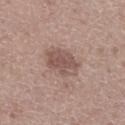Context: The subject is a male roughly 50 years of age. The total-body-photography lesion software estimated an area of roughly 10 mm², a shape eccentricity near 0.65, and a symmetry-axis asymmetry near 0.2. And it measured an average lesion color of about L≈52 a*≈18 b*≈22 (CIELAB), a lesion–skin lightness drop of about 10, and a normalized border contrast of about 7. The analysis additionally found a border-irregularity rating of about 2/10 and a peripheral color-asymmetry measure near 1. Captured under white-light illumination. Longest diameter approximately 4 mm. On the left lower leg. Cropped from a whole-body photographic skin survey; the tile spans about 15 mm.